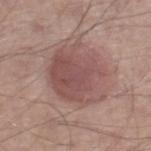The lesion was tiled from a total-body skin photograph and was not biopsied. A male subject in their mid-50s. The lesion is on the right lower leg. Cropped from a total-body skin-imaging series; the visible field is about 15 mm.